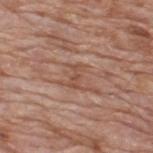Part of a total-body skin-imaging series; this lesion was reviewed on a skin check and was not flagged for biopsy.
A region of skin cropped from a whole-body photographic capture, roughly 15 mm wide.
A male subject aged 58 to 62.
Located on the upper back.